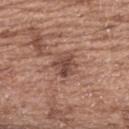{
  "biopsy_status": "not biopsied; imaged during a skin examination",
  "patient": {
    "sex": "female",
    "age_approx": 65
  },
  "site": "upper back",
  "image": {
    "source": "total-body photography crop",
    "field_of_view_mm": 15
  }
}